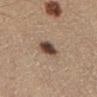The lesion was photographed on a routine skin check and not biopsied; there is no pathology result. The lesion-visualizer software estimated an area of roughly 5.5 mm², an outline eccentricity of about 0.55 (0 = round, 1 = elongated), and two-axis asymmetry of about 0.25. The analysis additionally found a border-irregularity rating of about 2/10, internal color variation of about 5.5 on a 0–10 scale, and a peripheral color-asymmetry measure near 2. The analysis additionally found a classifier nevus-likeness of about 100/100 and lesion-presence confidence of about 100/100. Imaged with cross-polarized lighting. The lesion is located on the left lower leg. The lesion's longest dimension is about 3 mm. A roughly 15 mm field-of-view crop from a total-body skin photograph. The subject is a male aged 38 to 42.